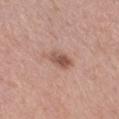Context:
Imaged with white-light lighting. Approximately 4 mm at its widest. A 15 mm close-up extracted from a 3D total-body photography capture. A female subject aged 53–57. The lesion is on the right thigh. Automated image analysis of the tile measured a lesion area of about 6.5 mm² and a symmetry-axis asymmetry near 0.3. The analysis additionally found a border-irregularity rating of about 3/10, a color-variation rating of about 4.5/10, and a peripheral color-asymmetry measure near 1.5.